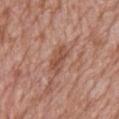Acquisition and patient details: The recorded lesion diameter is about 3.5 mm. The patient is a female aged 68–72. The lesion is on the head or neck. The total-body-photography lesion software estimated a footprint of about 6 mm², an eccentricity of roughly 0.85, and a symmetry-axis asymmetry near 0.25. It also reported a lesion color around L≈50 a*≈22 b*≈29 in CIELAB, a lesion–skin lightness drop of about 9, and a lesion-to-skin contrast of about 6.5 (normalized; higher = more distinct). A lesion tile, about 15 mm wide, cut from a 3D total-body photograph.